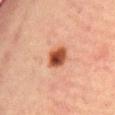Q: What are the patient's age and sex?
A: female, aged 38 to 42
Q: Lesion size?
A: about 3.5 mm
Q: What is the imaging modality?
A: ~15 mm crop, total-body skin-cancer survey
Q: How was the tile lit?
A: cross-polarized
Q: Where on the body is the lesion?
A: the abdomen